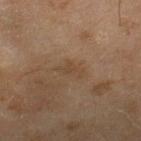No biopsy was performed on this lesion — it was imaged during a full skin examination and was not determined to be concerning.
The recorded lesion diameter is about 3.5 mm.
The lesion is on the leg.
The subject is a female about 60 years old.
This is a cross-polarized tile.
Cropped from a total-body skin-imaging series; the visible field is about 15 mm.
The lesion-visualizer software estimated a shape-asymmetry score of about 0.35 (0 = symmetric). And it measured an average lesion color of about L≈40 a*≈14 b*≈27 (CIELAB), a lesion–skin lightness drop of about 5, and a lesion-to-skin contrast of about 4.5 (normalized; higher = more distinct). And it measured border irregularity of about 4 on a 0–10 scale and peripheral color asymmetry of about 0. The analysis additionally found a nevus-likeness score of about 0/100.